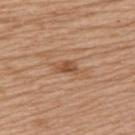workup: imaged on a skin check; not biopsied
illumination: white-light
patient: female, aged approximately 60
lesion diameter: about 2.5 mm
acquisition: ~15 mm crop, total-body skin-cancer survey
body site: the upper back